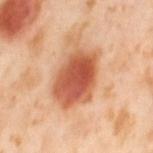workup = total-body-photography surveillance lesion; no biopsy
TBP lesion metrics = about 16 CIELAB-L* units darker than the surrounding skin; a border-irregularity rating of about 3.5/10, a color-variation rating of about 6.5/10, and radial color variation of about 2; an automated nevus-likeness rating near 100 out of 100 and a detector confidence of about 100 out of 100 that the crop contains a lesion
patient = female, aged approximately 55
body site = the left thigh
lighting = cross-polarized
acquisition = ~15 mm crop, total-body skin-cancer survey